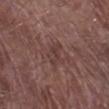biopsy_status: not biopsied; imaged during a skin examination
lesion_size:
  long_diameter_mm_approx: 3.0
image:
  source: total-body photography crop
  field_of_view_mm: 15
site: right lower leg
lighting: white-light
patient:
  sex: male
  age_approx: 80